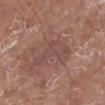Impression: Captured during whole-body skin photography for melanoma surveillance; the lesion was not biopsied.